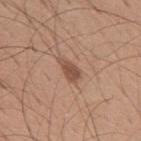workup=imaged on a skin check; not biopsied | body site=the mid back | acquisition=~15 mm crop, total-body skin-cancer survey | patient=male, aged 28–32.